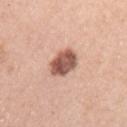{"biopsy_status": "not biopsied; imaged during a skin examination", "image": {"source": "total-body photography crop", "field_of_view_mm": 15}, "lighting": "white-light", "automated_metrics": {"area_mm2_approx": 8.0, "eccentricity": 0.6, "cielab_L": 55, "cielab_a": 23, "cielab_b": 27, "vs_skin_contrast_norm": 11.5, "border_irregularity_0_10": 1.5, "color_variation_0_10": 4.5, "peripheral_color_asymmetry": 1.5, "nevus_likeness_0_100": 35, "lesion_detection_confidence_0_100": 100}, "site": "arm", "patient": {"sex": "female", "age_approx": 60}, "lesion_size": {"long_diameter_mm_approx": 3.5}}